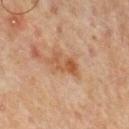Impression:
No biopsy was performed on this lesion — it was imaged during a full skin examination and was not determined to be concerning.
Image and clinical context:
A male patient, in their mid- to late 60s. The total-body-photography lesion software estimated a mean CIELAB color near L≈42 a*≈18 b*≈30 and a normalized lesion–skin contrast near 7.5. The software also gave a lesion-detection confidence of about 100/100. The tile uses cross-polarized illumination. The lesion is on the mid back. This image is a 15 mm lesion crop taken from a total-body photograph.